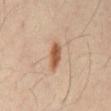  automated_metrics:
    nevus_likeness_0_100: 100
    lesion_detection_confidence_0_100: 100
  lighting: cross-polarized
  lesion_size:
    long_diameter_mm_approx: 3.5
  site: abdomen
  image:
    source: total-body photography crop
    field_of_view_mm: 15
  patient:
    sex: male
    age_approx: 40